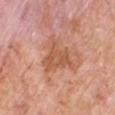Notes:
– workup — imaged on a skin check; not biopsied
– automated lesion analysis — an average lesion color of about L≈56 a*≈25 b*≈34 (CIELAB), about 8 CIELAB-L* units darker than the surrounding skin, and a normalized border contrast of about 6.5; lesion-presence confidence of about 100/100
– subject — male, in their 60s
– lesion size — ~5 mm (longest diameter)
– illumination — white-light
– acquisition — ~15 mm crop, total-body skin-cancer survey
– location — the right upper arm